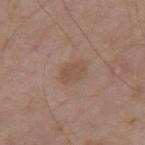Q: Was this lesion biopsied?
A: catalogued during a skin exam; not biopsied
Q: How large is the lesion?
A: ≈3 mm
Q: How was this image acquired?
A: ~15 mm crop, total-body skin-cancer survey
Q: How was the tile lit?
A: white-light illumination
Q: Lesion location?
A: the upper back
Q: Patient demographics?
A: male, about 70 years old